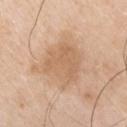The lesion was photographed on a routine skin check and not biopsied; there is no pathology result. Automated tile analysis of the lesion measured a lesion area of about 17 mm², an outline eccentricity of about 0.45 (0 = round, 1 = elongated), and a shape-asymmetry score of about 0.3 (0 = symmetric). A male subject, aged 78–82. From the chest. A roughly 15 mm field-of-view crop from a total-body skin photograph. Approximately 5 mm at its widest.